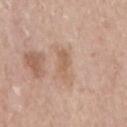<case>
  <biopsy_status>not biopsied; imaged during a skin examination</biopsy_status>
  <image>
    <source>total-body photography crop</source>
    <field_of_view_mm>15</field_of_view_mm>
  </image>
  <lesion_size>
    <long_diameter_mm_approx>3.5</long_diameter_mm_approx>
  </lesion_size>
  <site>right upper arm</site>
  <lighting>white-light</lighting>
  <patient>
    <sex>female</sex>
    <age_approx>60</age_approx>
  </patient>
</case>